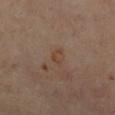Assessment: The lesion was tiled from a total-body skin photograph and was not biopsied.